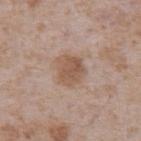This lesion was catalogued during total-body skin photography and was not selected for biopsy.
Longest diameter approximately 3.5 mm.
A male subject in their mid- to late 60s.
On the abdomen.
A 15 mm close-up extracted from a 3D total-body photography capture.